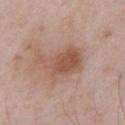Clinical impression: The lesion was tiled from a total-body skin photograph and was not biopsied. Image and clinical context: From the front of the torso. A male patient, about 60 years old. This is a white-light tile. A lesion tile, about 15 mm wide, cut from a 3D total-body photograph. Longest diameter approximately 5.5 mm. The total-body-photography lesion software estimated a footprint of about 15 mm², an eccentricity of roughly 0.8, and two-axis asymmetry of about 0.35. The analysis additionally found radial color variation of about 1.5. The software also gave an automated nevus-likeness rating near 45 out of 100.